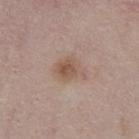Recorded during total-body skin imaging; not selected for excision or biopsy. Automated image analysis of the tile measured a footprint of about 7.5 mm², an outline eccentricity of about 0.65 (0 = round, 1 = elongated), and two-axis asymmetry of about 0.25. The analysis additionally found an average lesion color of about L≈54 a*≈17 b*≈27 (CIELAB) and roughly 8 lightness units darker than nearby skin. The software also gave a border-irregularity rating of about 2.5/10, internal color variation of about 4 on a 0–10 scale, and radial color variation of about 1.5. The software also gave a nevus-likeness score of about 45/100 and a detector confidence of about 100 out of 100 that the crop contains a lesion. A male patient, aged approximately 65. The recorded lesion diameter is about 3.5 mm. Captured under white-light illumination. A lesion tile, about 15 mm wide, cut from a 3D total-body photograph. On the chest.